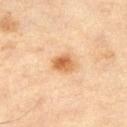Q: Illumination type?
A: cross-polarized illumination
Q: How was this image acquired?
A: ~15 mm crop, total-body skin-cancer survey
Q: Who is the patient?
A: male, roughly 60 years of age
Q: What is the anatomic site?
A: the leg